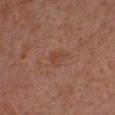biopsy_status: not biopsied; imaged during a skin examination
patient:
  sex: male
  age_approx: 30
image:
  source: total-body photography crop
  field_of_view_mm: 15
site: left upper arm